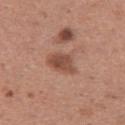{"biopsy_status": "not biopsied; imaged during a skin examination", "lesion_size": {"long_diameter_mm_approx": 3.5}, "patient": {"sex": "female", "age_approx": 30}, "image": {"source": "total-body photography crop", "field_of_view_mm": 15}, "site": "right thigh", "automated_metrics": {"vs_skin_darker_L": 11.0, "vs_skin_contrast_norm": 7.5, "nevus_likeness_0_100": 45}}